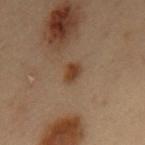site: mid back
image:
  source: total-body photography crop
  field_of_view_mm: 15
patient:
  sex: male
  age_approx: 55
lesion_size:
  long_diameter_mm_approx: 2.5